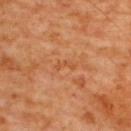Part of a total-body skin-imaging series; this lesion was reviewed on a skin check and was not flagged for biopsy.